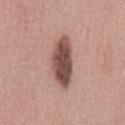This image is a 15 mm lesion crop taken from a total-body photograph.
A female subject, approximately 40 years of age.
The total-body-photography lesion software estimated border irregularity of about 2 on a 0–10 scale, a color-variation rating of about 6/10, and radial color variation of about 2.
The lesion is located on the lower back.